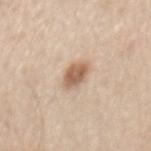Imaged during a routine full-body skin examination; the lesion was not biopsied and no histopathology is available. The lesion is on the back. Longest diameter approximately 3 mm. The patient is a male about 60 years old. This image is a 15 mm lesion crop taken from a total-body photograph.